A 15 mm close-up extracted from a 3D total-body photography capture. The lesion is located on the back. The subject is a male approximately 45 years of age: 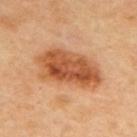Q: Automated lesion metrics?
A: a lesion color around L≈53 a*≈26 b*≈38 in CIELAB, a lesion–skin lightness drop of about 14, and a normalized lesion–skin contrast near 9.5; a border-irregularity index near 2.5/10 and peripheral color asymmetry of about 2.5; an automated nevus-likeness rating near 100 out of 100 and lesion-presence confidence of about 100/100
Q: What lighting was used for the tile?
A: cross-polarized illumination
Q: What did pathology find?
A: a seborrheic keratosis (benign)